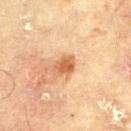notes = total-body-photography surveillance lesion; no biopsy | diameter = ≈2.5 mm | illumination = cross-polarized | subject = male, approximately 70 years of age | body site = the left thigh | acquisition = ~15 mm tile from a whole-body skin photo.